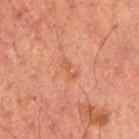| field | value |
|---|---|
| workup | no biopsy performed (imaged during a skin exam) |
| tile lighting | cross-polarized illumination |
| subject | male, roughly 50 years of age |
| image | 15 mm crop, total-body photography |
| lesion diameter | about 2.5 mm |
| body site | the right upper arm |